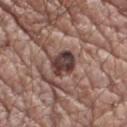| key | value |
|---|---|
| biopsy status | no biopsy performed (imaged during a skin exam) |
| size | ~3 mm (longest diameter) |
| patient | male, roughly 65 years of age |
| image source | ~15 mm tile from a whole-body skin photo |
| site | the right upper arm |